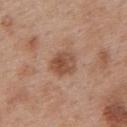Clinical impression:
The lesion was tiled from a total-body skin photograph and was not biopsied.
Background:
The subject is a female approximately 40 years of age. Cropped from a whole-body photographic skin survey; the tile spans about 15 mm. The lesion is on the upper back. The lesion's longest dimension is about 3 mm.